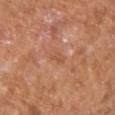The lesion was tiled from a total-body skin photograph and was not biopsied. The recorded lesion diameter is about 2.5 mm. From the left upper arm. The patient is a male approximately 75 years of age. Imaged with white-light lighting. This image is a 15 mm lesion crop taken from a total-body photograph.